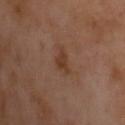notes: imaged on a skin check; not biopsied | anatomic site: the chest | automated metrics: an area of roughly 4 mm², a shape eccentricity near 0.55, and two-axis asymmetry of about 0.4; a border-irregularity index near 4/10, a color-variation rating of about 2.5/10, and peripheral color asymmetry of about 1; a classifier nevus-likeness of about 35/100 | lesion size: ~2.5 mm (longest diameter) | subject: male, approximately 55 years of age | image source: 15 mm crop, total-body photography | tile lighting: cross-polarized.